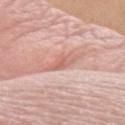{
  "biopsy_status": "not biopsied; imaged during a skin examination",
  "image": {
    "source": "total-body photography crop",
    "field_of_view_mm": 15
  },
  "patient": {
    "sex": "female",
    "age_approx": 50
  },
  "lesion_size": {
    "long_diameter_mm_approx": 3.5
  },
  "site": "left forearm",
  "automated_metrics": {
    "area_mm2_approx": 4.5,
    "eccentricity": 0.85,
    "shape_asymmetry": 0.35,
    "cielab_L": 63,
    "cielab_a": 25,
    "cielab_b": 27,
    "vs_skin_darker_L": 9.0,
    "vs_skin_contrast_norm": 5.5,
    "border_irregularity_0_10": 4.0,
    "color_variation_0_10": 3.5,
    "peripheral_color_asymmetry": 1.0
  }
}